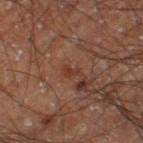Impression: Captured during whole-body skin photography for melanoma surveillance; the lesion was not biopsied.